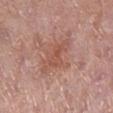Recorded during total-body skin imaging; not selected for excision or biopsy. A female subject, aged 73–77. The recorded lesion diameter is about 3 mm. Captured under white-light illumination. The lesion is located on the right lower leg. Cropped from a total-body skin-imaging series; the visible field is about 15 mm.